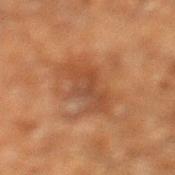Recorded during total-body skin imaging; not selected for excision or biopsy.
The subject is a male about 60 years old.
Cropped from a total-body skin-imaging series; the visible field is about 15 mm.
Automated tile analysis of the lesion measured a nevus-likeness score of about 0/100 and lesion-presence confidence of about 100/100.
From the left lower leg.